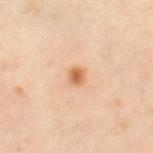follow-up: catalogued during a skin exam; not biopsied | site: the leg | subject: female, aged approximately 40 | illumination: cross-polarized illumination | image: ~15 mm tile from a whole-body skin photo.